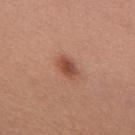Case summary:
* biopsy status: imaged on a skin check; not biopsied
* diameter: about 3 mm
* anatomic site: the upper back
* imaging modality: total-body-photography crop, ~15 mm field of view
* patient: female, in their 50s